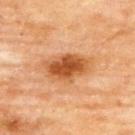Findings:
• follow-up · no biopsy performed (imaged during a skin exam)
• diameter · ~5.5 mm (longest diameter)
• location · the upper back
• patient · female, aged approximately 80
• image source · 15 mm crop, total-body photography
• automated lesion analysis · border irregularity of about 3 on a 0–10 scale, internal color variation of about 5 on a 0–10 scale, and a peripheral color-asymmetry measure near 1.5